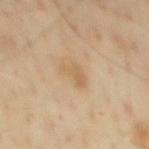No biopsy was performed on this lesion — it was imaged during a full skin examination and was not determined to be concerning.
The patient is a male approximately 55 years of age.
The lesion-visualizer software estimated an area of roughly 4.5 mm², an eccentricity of roughly 0.75, and two-axis asymmetry of about 0.5. The analysis additionally found a border-irregularity rating of about 5/10, internal color variation of about 1.5 on a 0–10 scale, and a peripheral color-asymmetry measure near 0.5.
Located on the mid back.
Longest diameter approximately 3 mm.
Imaged with cross-polarized lighting.
A 15 mm close-up tile from a total-body photography series done for melanoma screening.